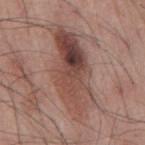Assessment: Recorded during total-body skin imaging; not selected for excision or biopsy. Acquisition and patient details: The lesion's longest dimension is about 10 mm. A male subject aged 53 to 57. Located on the mid back. Cropped from a total-body skin-imaging series; the visible field is about 15 mm. Automated image analysis of the tile measured a border-irregularity rating of about 5/10 and a color-variation rating of about 10/10. Captured under white-light illumination.